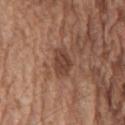workup: no biopsy performed (imaged during a skin exam); illumination: white-light illumination; image source: ~15 mm tile from a whole-body skin photo; size: ≈3.5 mm; body site: the chest; subject: male, aged 73–77.